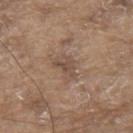Part of a total-body skin-imaging series; this lesion was reviewed on a skin check and was not flagged for biopsy.
The patient is a male in their 80s.
A 15 mm close-up extracted from a 3D total-body photography capture.
Imaged with white-light lighting.
The total-body-photography lesion software estimated a lesion color around L≈49 a*≈16 b*≈25 in CIELAB and a lesion-to-skin contrast of about 6 (normalized; higher = more distinct).
Measured at roughly 3.5 mm in maximum diameter.
On the upper back.